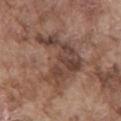notes = imaged on a skin check; not biopsied | subject = male, aged around 75 | acquisition = ~15 mm tile from a whole-body skin photo | site = the mid back | size = ≈8 mm | TBP lesion metrics = a symmetry-axis asymmetry near 0.65; a nevus-likeness score of about 10/100 and a lesion-detection confidence of about 90/100.